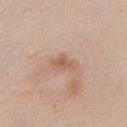Assessment: Imaged during a routine full-body skin examination; the lesion was not biopsied and no histopathology is available.